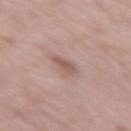workup: catalogued during a skin exam; not biopsied
image source: ~15 mm crop, total-body skin-cancer survey
lighting: white-light illumination
location: the right thigh
patient: male, aged approximately 70
TBP lesion metrics: a lesion area of about 4 mm², an outline eccentricity of about 0.8 (0 = round, 1 = elongated), and a shape-asymmetry score of about 0.4 (0 = symmetric); a border-irregularity rating of about 4/10, a within-lesion color-variation index near 2/10, and a peripheral color-asymmetry measure near 0.5